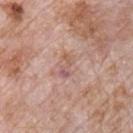Captured during whole-body skin photography for melanoma surveillance; the lesion was not biopsied. A region of skin cropped from a whole-body photographic capture, roughly 15 mm wide. The patient is a male aged around 70. From the chest.